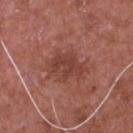The lesion was photographed on a routine skin check and not biopsied; there is no pathology result. On the chest. Cropped from a whole-body photographic skin survey; the tile spans about 15 mm. The patient is a male aged 63 to 67.